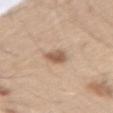<lesion>
<patient>
  <sex>male</sex>
  <age_approx>60</age_approx>
</patient>
<site>back</site>
<automated_metrics>
  <cielab_L>57</cielab_L>
  <cielab_a>18</cielab_a>
  <cielab_b>30</cielab_b>
  <vs_skin_darker_L>12.0</vs_skin_darker_L>
  <vs_skin_contrast_norm>8.0</vs_skin_contrast_norm>
</automated_metrics>
<lesion_size>
  <long_diameter_mm_approx>3.0</long_diameter_mm_approx>
</lesion_size>
<image>
  <source>total-body photography crop</source>
  <field_of_view_mm>15</field_of_view_mm>
</image>
</lesion>